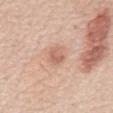workup: imaged on a skin check; not biopsied | image source: ~15 mm tile from a whole-body skin photo | lighting: white-light | lesion size: about 2.5 mm | image-analysis metrics: a mean CIELAB color near L≈62 a*≈23 b*≈29 and a lesion–skin lightness drop of about 9; a detector confidence of about 100 out of 100 that the crop contains a lesion | patient: male, roughly 70 years of age | anatomic site: the mid back.